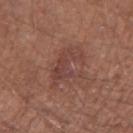patient: male, aged approximately 55
image: ~15 mm tile from a whole-body skin photo
anatomic site: the right forearm
lesion diameter: ≈5 mm
lighting: white-light illumination